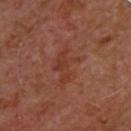Q: What are the patient's age and sex?
A: male, roughly 50 years of age
Q: What is the anatomic site?
A: the upper back
Q: Lesion size?
A: ≈5 mm
Q: What is the imaging modality?
A: 15 mm crop, total-body photography
Q: Illumination type?
A: cross-polarized illumination